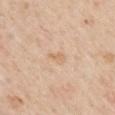Imaged during a routine full-body skin examination; the lesion was not biopsied and no histopathology is available. Measured at roughly 2.5 mm in maximum diameter. The lesion is located on the mid back. A male patient, aged approximately 65. Imaged with white-light lighting. A 15 mm close-up extracted from a 3D total-body photography capture. The total-body-photography lesion software estimated an area of roughly 2.5 mm².